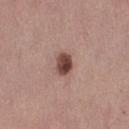Findings:
• notes: catalogued during a skin exam; not biopsied
• patient: female, about 50 years old
• location: the front of the torso
• lighting: white-light
• image source: ~15 mm tile from a whole-body skin photo
• size: ~2.5 mm (longest diameter)